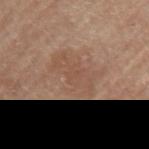follow-up: imaged on a skin check; not biopsied
site: the left upper arm
illumination: white-light illumination
image: 15 mm crop, total-body photography
diameter: ~5.5 mm (longest diameter)
patient: female, aged approximately 75
automated lesion analysis: a lesion area of about 15 mm² and an outline eccentricity of about 0.8 (0 = round, 1 = elongated); a lesion color around L≈52 a*≈18 b*≈28 in CIELAB and a lesion-to-skin contrast of about 4.5 (normalized; higher = more distinct); border irregularity of about 4.5 on a 0–10 scale, a within-lesion color-variation index near 2.5/10, and peripheral color asymmetry of about 0.5; a nevus-likeness score of about 0/100 and lesion-presence confidence of about 100/100